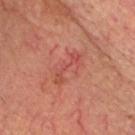Case summary:
– acquisition: ~15 mm tile from a whole-body skin photo
– lesion size: ≈4.5 mm
– tile lighting: cross-polarized
– subject: male, approximately 55 years of age
– body site: the head or neck